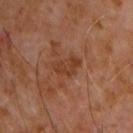Imaged during a routine full-body skin examination; the lesion was not biopsied and no histopathology is available. The subject is a male aged 58 to 62. Cropped from a whole-body photographic skin survey; the tile spans about 15 mm. The lesion is located on the chest.